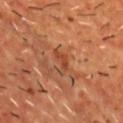biopsy status = no biopsy performed (imaged during a skin exam) | tile lighting = cross-polarized | imaging modality = 15 mm crop, total-body photography | patient = male, in their mid- to late 50s | size = ~3 mm (longest diameter) | location = the chest | automated metrics = a detector confidence of about 100 out of 100 that the crop contains a lesion.